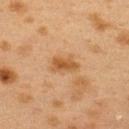notes = catalogued during a skin exam; not biopsied
site = the upper back
subject = female, in their 40s
image = 15 mm crop, total-body photography
lighting = cross-polarized